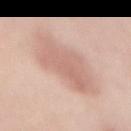Assessment: Imaged during a routine full-body skin examination; the lesion was not biopsied and no histopathology is available. Clinical summary: The subject is a female aged approximately 50. The tile uses white-light illumination. Cropped from a total-body skin-imaging series; the visible field is about 15 mm. On the abdomen.